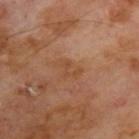Imaged during a routine full-body skin examination; the lesion was not biopsied and no histopathology is available. Located on the upper back. A male subject aged around 70. A 15 mm close-up extracted from a 3D total-body photography capture.